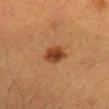Q: Is there a histopathology result?
A: no biopsy performed (imaged during a skin exam)
Q: What are the patient's age and sex?
A: female, aged around 40
Q: What kind of image is this?
A: ~15 mm crop, total-body skin-cancer survey
Q: How was the tile lit?
A: cross-polarized illumination
Q: Lesion location?
A: the head or neck
Q: How large is the lesion?
A: ≈3.5 mm